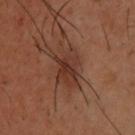<case>
  <biopsy_status>not biopsied; imaged during a skin examination</biopsy_status>
  <site>upper back</site>
  <image>
    <source>total-body photography crop</source>
    <field_of_view_mm>15</field_of_view_mm>
  </image>
  <lighting>cross-polarized</lighting>
  <automated_metrics>
    <area_mm2_approx>13.0</area_mm2_approx>
    <eccentricity>0.8</eccentricity>
    <cielab_L>34</cielab_L>
    <cielab_a>21</cielab_a>
    <cielab_b>26</cielab_b>
    <vs_skin_darker_L>8.0</vs_skin_darker_L>
    <nevus_likeness_0_100>15</nevus_likeness_0_100>
    <lesion_detection_confidence_0_100>100</lesion_detection_confidence_0_100>
  </automated_metrics>
  <patient>
    <sex>male</sex>
    <age_approx>40</age_approx>
  </patient>
</case>